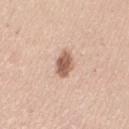biopsy status — imaged on a skin check; not biopsied | site — the left upper arm | imaging modality — 15 mm crop, total-body photography | lesion diameter — ≈4 mm | subject — female, in their mid-40s.